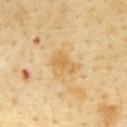Q: Was a biopsy performed?
A: total-body-photography surveillance lesion; no biopsy
Q: What is the anatomic site?
A: the chest
Q: What is the imaging modality?
A: ~15 mm tile from a whole-body skin photo
Q: What lighting was used for the tile?
A: cross-polarized illumination
Q: Who is the patient?
A: male, in their mid-60s